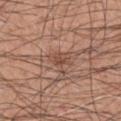Background: Located on the left upper arm. A lesion tile, about 15 mm wide, cut from a 3D total-body photograph. The patient is a male aged 58 to 62.